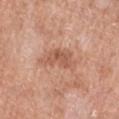<lesion>
<biopsy_status>not biopsied; imaged during a skin examination</biopsy_status>
<image>
  <source>total-body photography crop</source>
  <field_of_view_mm>15</field_of_view_mm>
</image>
<patient>
  <sex>female</sex>
  <age_approx>70</age_approx>
</patient>
<automated_metrics>
  <eccentricity>0.9</eccentricity>
  <shape_asymmetry>0.45</shape_asymmetry>
  <vs_skin_darker_L>9.0</vs_skin_darker_L>
  <vs_skin_contrast_norm>6.5</vs_skin_contrast_norm>
  <border_irregularity_0_10>5.0</border_irregularity_0_10>
  <color_variation_0_10>3.0</color_variation_0_10>
  <peripheral_color_asymmetry>1.0</peripheral_color_asymmetry>
  <nevus_likeness_0_100>0</nevus_likeness_0_100>
</automated_metrics>
<site>left lower leg</site>
<lesion_size>
  <long_diameter_mm_approx>5.5</long_diameter_mm_approx>
</lesion_size>
</lesion>